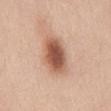No biopsy was performed on this lesion — it was imaged during a full skin examination and was not determined to be concerning.
From the abdomen.
Imaged with white-light lighting.
The subject is a female in their mid- to late 30s.
Automated image analysis of the tile measured an area of roughly 13 mm² and two-axis asymmetry of about 0.15.
Cropped from a whole-body photographic skin survey; the tile spans about 15 mm.